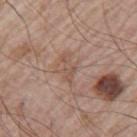Image and clinical context:
The subject is a male aged 63 to 67. A lesion tile, about 15 mm wide, cut from a 3D total-body photograph. Captured under white-light illumination. Located on the left upper arm. Measured at roughly 3.5 mm in maximum diameter.